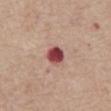Part of a total-body skin-imaging series; this lesion was reviewed on a skin check and was not flagged for biopsy. This is a white-light tile. A male patient, aged 63 to 67. Located on the abdomen. Longest diameter approximately 2.5 mm. A 15 mm crop from a total-body photograph taken for skin-cancer surveillance.